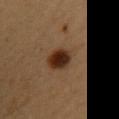Assessment: The lesion was tiled from a total-body skin photograph and was not biopsied. Image and clinical context: A roughly 15 mm field-of-view crop from a total-body skin photograph. The tile uses cross-polarized illumination. The recorded lesion diameter is about 3.5 mm. An algorithmic analysis of the crop reported an area of roughly 7.5 mm². The software also gave a lesion color around L≈26 a*≈18 b*≈26 in CIELAB, a lesion–skin lightness drop of about 13, and a lesion-to-skin contrast of about 13.5 (normalized; higher = more distinct). The patient is a female approximately 30 years of age. From the upper back.